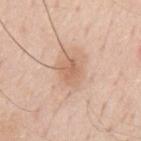Imaged during a routine full-body skin examination; the lesion was not biopsied and no histopathology is available.
The lesion is located on the mid back.
Cropped from a whole-body photographic skin survey; the tile spans about 15 mm.
This is a white-light tile.
Automated image analysis of the tile measured an area of roughly 5 mm², an eccentricity of roughly 0.65, and a symmetry-axis asymmetry near 0.2. It also reported a lesion-to-skin contrast of about 6 (normalized; higher = more distinct). And it measured border irregularity of about 2 on a 0–10 scale and internal color variation of about 2.5 on a 0–10 scale. The software also gave a nevus-likeness score of about 35/100 and lesion-presence confidence of about 100/100.
Approximately 3 mm at its widest.
A male subject aged approximately 60.